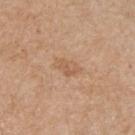| feature | finding |
|---|---|
| workup | catalogued during a skin exam; not biopsied |
| illumination | white-light illumination |
| image-analysis metrics | an eccentricity of roughly 0.85 and two-axis asymmetry of about 0.25; a mean CIELAB color near L≈58 a*≈19 b*≈34, a lesion–skin lightness drop of about 7, and a lesion-to-skin contrast of about 5 (normalized; higher = more distinct); a classifier nevus-likeness of about 0/100 and a lesion-detection confidence of about 100/100 |
| acquisition | ~15 mm tile from a whole-body skin photo |
| anatomic site | the chest |
| lesion diameter | about 3.5 mm |
| subject | male, about 70 years old |